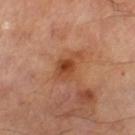Q: Was this lesion biopsied?
A: total-body-photography surveillance lesion; no biopsy
Q: Automated lesion metrics?
A: a classifier nevus-likeness of about 15/100
Q: Where on the body is the lesion?
A: the left thigh
Q: What is the lesion's diameter?
A: ≈4 mm
Q: What lighting was used for the tile?
A: cross-polarized illumination
Q: What is the imaging modality?
A: total-body-photography crop, ~15 mm field of view
Q: What are the patient's age and sex?
A: male, about 70 years old